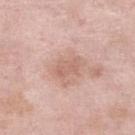Q: Was this lesion biopsied?
A: total-body-photography surveillance lesion; no biopsy
Q: What kind of image is this?
A: ~15 mm crop, total-body skin-cancer survey
Q: Who is the patient?
A: female, aged around 70
Q: What is the lesion's diameter?
A: ≈3 mm
Q: What did automated image analysis measure?
A: an area of roughly 4.5 mm², an outline eccentricity of about 0.85 (0 = round, 1 = elongated), and a shape-asymmetry score of about 0.3 (0 = symmetric); a lesion–skin lightness drop of about 8 and a normalized border contrast of about 5; a border-irregularity index near 3/10, a within-lesion color-variation index near 1.5/10, and radial color variation of about 0.5
Q: Lesion location?
A: the right lower leg
Q: Illumination type?
A: white-light